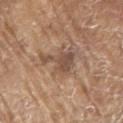The lesion was photographed on a routine skin check and not biopsied; there is no pathology result. The lesion is located on the right upper arm. The patient is a male in their 80s. Approximately 5 mm at its widest. Captured under white-light illumination. A 15 mm close-up tile from a total-body photography series done for melanoma screening.